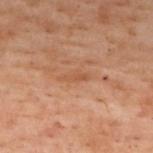Part of a total-body skin-imaging series; this lesion was reviewed on a skin check and was not flagged for biopsy. This is a cross-polarized tile. The lesion is located on the back. The total-body-photography lesion software estimated an eccentricity of roughly 0.9 and two-axis asymmetry of about 0.45. It also reported border irregularity of about 4.5 on a 0–10 scale and a color-variation rating of about 0.5/10. And it measured an automated nevus-likeness rating near 0 out of 100. About 3 mm across. The subject is a female in their mid- to late 50s. A lesion tile, about 15 mm wide, cut from a 3D total-body photograph.